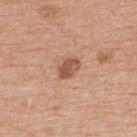biopsy status: no biopsy performed (imaged during a skin exam) | patient: female, in their mid-60s | illumination: white-light | location: the upper back | size: ~3 mm (longest diameter) | acquisition: 15 mm crop, total-body photography.